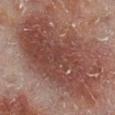{"biopsy_status": "not biopsied; imaged during a skin examination", "automated_metrics": {"cielab_L": 38, "cielab_a": 20, "cielab_b": 22, "vs_skin_darker_L": 9.0, "vs_skin_contrast_norm": 8.0}, "image": {"source": "total-body photography crop", "field_of_view_mm": 15}, "lesion_size": {"long_diameter_mm_approx": 12.0}, "site": "right lower leg", "patient": {"sex": "male", "age_approx": 60}, "lighting": "cross-polarized"}